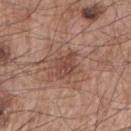automated metrics: an area of roughly 6 mm², an eccentricity of roughly 0.7, and a symmetry-axis asymmetry near 0.2 | size: ~3 mm (longest diameter) | image: 15 mm crop, total-body photography | site: the right upper arm | subject: male, in their mid-60s.